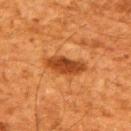The lesion was tiled from a total-body skin photograph and was not biopsied.
On the back.
The subject is a male roughly 60 years of age.
Cropped from a total-body skin-imaging series; the visible field is about 15 mm.